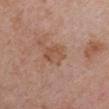Clinical impression: This lesion was catalogued during total-body skin photography and was not selected for biopsy. Background: Automated tile analysis of the lesion measured an eccentricity of roughly 0.55 and a shape-asymmetry score of about 0.2 (0 = symmetric). The software also gave a mean CIELAB color near L≈52 a*≈20 b*≈30 and roughly 7 lightness units darker than nearby skin. The analysis additionally found lesion-presence confidence of about 100/100. About 3 mm across. A 15 mm close-up extracted from a 3D total-body photography capture. A female patient, aged approximately 45. From the right upper arm.